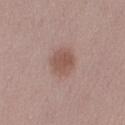Q: Is there a histopathology result?
A: no biopsy performed (imaged during a skin exam)
Q: Illumination type?
A: white-light illumination
Q: What kind of image is this?
A: total-body-photography crop, ~15 mm field of view
Q: Automated lesion metrics?
A: a lesion area of about 8.5 mm², an outline eccentricity of about 0.6 (0 = round, 1 = elongated), and a shape-asymmetry score of about 0.15 (0 = symmetric); a mean CIELAB color near L≈53 a*≈19 b*≈24, a lesion–skin lightness drop of about 9, and a normalized lesion–skin contrast near 7; a detector confidence of about 100 out of 100 that the crop contains a lesion
Q: Lesion location?
A: the right upper arm
Q: Lesion size?
A: ~4 mm (longest diameter)
Q: What are the patient's age and sex?
A: female, in their mid- to late 40s